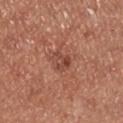No biopsy was performed on this lesion — it was imaged during a full skin examination and was not determined to be concerning. The patient is a male aged 68 to 72. The lesion is located on the right lower leg. This is a white-light tile. A 15 mm close-up extracted from a 3D total-body photography capture.